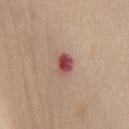Clinical summary:
From the front of the torso. Imaged with white-light lighting. A female patient, aged around 40. Approximately 2.5 mm at its widest. An algorithmic analysis of the crop reported a footprint of about 4 mm² and an outline eccentricity of about 0.7 (0 = round, 1 = elongated). The software also gave a lesion-to-skin contrast of about 11 (normalized; higher = more distinct). Cropped from a total-body skin-imaging series; the visible field is about 15 mm.